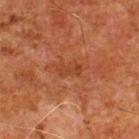{"biopsy_status": "not biopsied; imaged during a skin examination", "image": {"source": "total-body photography crop", "field_of_view_mm": 15}, "lighting": "cross-polarized", "patient": {"sex": "male", "age_approx": 80}, "lesion_size": {"long_diameter_mm_approx": 3.0}, "site": "upper back"}